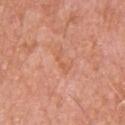The lesion was photographed on a routine skin check and not biopsied; there is no pathology result.
Located on the upper back.
Automated image analysis of the tile measured a mean CIELAB color near L≈60 a*≈27 b*≈35, about 6 CIELAB-L* units darker than the surrounding skin, and a normalized lesion–skin contrast near 4.5. The software also gave peripheral color asymmetry of about 0.
Captured under white-light illumination.
Measured at roughly 2.5 mm in maximum diameter.
A male subject, aged 53 to 57.
A lesion tile, about 15 mm wide, cut from a 3D total-body photograph.